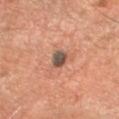Imaged during a routine full-body skin examination; the lesion was not biopsied and no histopathology is available. Imaged with cross-polarized lighting. Approximately 2.5 mm at its widest. From the arm. A 15 mm crop from a total-body photograph taken for skin-cancer surveillance. The patient is a male aged 53 to 57.